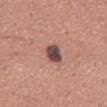Impression: This lesion was catalogued during total-body skin photography and was not selected for biopsy.